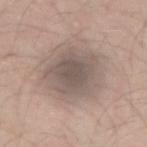Q: Illumination type?
A: white-light
Q: What is the lesion's diameter?
A: about 6.5 mm
Q: Patient demographics?
A: male, in their mid-30s
Q: What did automated image analysis measure?
A: an area of roughly 24 mm², a shape eccentricity near 0.65, and two-axis asymmetry of about 0.2; a border-irregularity rating of about 2/10 and peripheral color asymmetry of about 1; a nevus-likeness score of about 20/100 and lesion-presence confidence of about 55/100
Q: What kind of image is this?
A: ~15 mm tile from a whole-body skin photo
Q: What is the anatomic site?
A: the right forearm